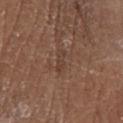Notes:
– biopsy status: catalogued during a skin exam; not biopsied
– lesion diameter: ~2.5 mm (longest diameter)
– patient: female, about 75 years old
– tile lighting: white-light illumination
– site: the right lower leg
– image source: 15 mm crop, total-body photography